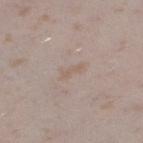Q: Was a biopsy performed?
A: imaged on a skin check; not biopsied
Q: What is the imaging modality?
A: total-body-photography crop, ~15 mm field of view
Q: Patient demographics?
A: female, aged 23–27
Q: What is the lesion's diameter?
A: about 2.5 mm
Q: Where on the body is the lesion?
A: the left thigh